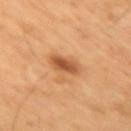Impression: No biopsy was performed on this lesion — it was imaged during a full skin examination and was not determined to be concerning. Image and clinical context: A male patient in their 60s. This image is a 15 mm lesion crop taken from a total-body photograph. The lesion is located on the right upper arm. The lesion-visualizer software estimated internal color variation of about 4 on a 0–10 scale and a peripheral color-asymmetry measure near 1.5. And it measured a classifier nevus-likeness of about 90/100.